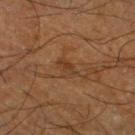  biopsy_status: not biopsied; imaged during a skin examination
  site: leg
  patient:
    sex: male
    age_approx: 65
  lighting: cross-polarized
  lesion_size:
    long_diameter_mm_approx: 3.0
  image:
    source: total-body photography crop
    field_of_view_mm: 15
  automated_metrics:
    area_mm2_approx: 3.5
    eccentricity: 0.9
    shape_asymmetry: 0.3
    cielab_L: 30
    cielab_a: 17
    cielab_b: 26
    vs_skin_darker_L: 6.0
    vs_skin_contrast_norm: 6.0